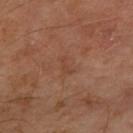workup: total-body-photography surveillance lesion; no biopsy | lesion diameter: ≈2.5 mm | patient: male, aged 58 to 62 | tile lighting: cross-polarized | anatomic site: the left forearm | image source: 15 mm crop, total-body photography.